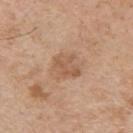Findings:
– follow-up — no biopsy performed (imaged during a skin exam)
– image source — total-body-photography crop, ~15 mm field of view
– patient — male, aged 58 to 62
– location — the upper back
– TBP lesion metrics — a lesion color around L≈57 a*≈19 b*≈32 in CIELAB, roughly 8 lightness units darker than nearby skin, and a normalized lesion–skin contrast near 5.5; a lesion-detection confidence of about 100/100
– lesion diameter — ≈3.5 mm